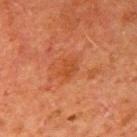{
  "biopsy_status": "not biopsied; imaged during a skin examination",
  "image": {
    "source": "total-body photography crop",
    "field_of_view_mm": 15
  },
  "site": "right upper arm",
  "lighting": "cross-polarized",
  "lesion_size": {
    "long_diameter_mm_approx": 2.5
  },
  "patient": {
    "sex": "male",
    "age_approx": 60
  }
}